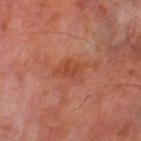Clinical impression: Imaged during a routine full-body skin examination; the lesion was not biopsied and no histopathology is available. Acquisition and patient details: A close-up tile cropped from a whole-body skin photograph, about 15 mm across. The recorded lesion diameter is about 3 mm. The tile uses cross-polarized illumination. From the left thigh. The subject is a male about 70 years old.